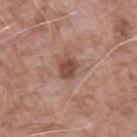{
  "biopsy_status": "not biopsied; imaged during a skin examination",
  "patient": {
    "sex": "male",
    "age_approx": 75
  },
  "automated_metrics": {
    "area_mm2_approx": 4.0,
    "eccentricity": 0.55,
    "shape_asymmetry": 0.2,
    "cielab_L": 47,
    "cielab_a": 22,
    "cielab_b": 27,
    "vs_skin_darker_L": 12.0,
    "vs_skin_contrast_norm": 8.5,
    "border_irregularity_0_10": 2.0,
    "color_variation_0_10": 2.0,
    "peripheral_color_asymmetry": 0.5,
    "nevus_likeness_0_100": 10,
    "lesion_detection_confidence_0_100": 100
  },
  "image": {
    "source": "total-body photography crop",
    "field_of_view_mm": 15
  },
  "lighting": "white-light",
  "lesion_size": {
    "long_diameter_mm_approx": 2.5
  },
  "site": "arm"
}